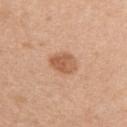workup: total-body-photography surveillance lesion; no biopsy | acquisition: 15 mm crop, total-body photography | body site: the right upper arm | patient: female, about 40 years old | lesion size: ≈3.5 mm | automated metrics: a footprint of about 7 mm² and a symmetry-axis asymmetry near 0.15; a mean CIELAB color near L≈58 a*≈23 b*≈34, a lesion–skin lightness drop of about 11, and a normalized border contrast of about 7.5; a border-irregularity index near 1.5/10, a color-variation rating of about 4/10, and radial color variation of about 1.5; an automated nevus-likeness rating near 75 out of 100 and a lesion-detection confidence of about 100/100.